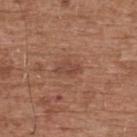Context:
Cropped from a total-body skin-imaging series; the visible field is about 15 mm. Automated tile analysis of the lesion measured a lesion area of about 4 mm² and a shape eccentricity near 0.8. The analysis additionally found a nevus-likeness score of about 5/100 and a lesion-detection confidence of about 100/100. From the upper back. Captured under white-light illumination. The subject is a male approximately 75 years of age. The lesion's longest dimension is about 3 mm.